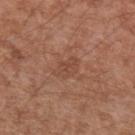Q: What are the patient's age and sex?
A: male, aged 53 to 57
Q: How was this image acquired?
A: 15 mm crop, total-body photography
Q: What did automated image analysis measure?
A: a mean CIELAB color near L≈46 a*≈22 b*≈29 and a normalized lesion–skin contrast near 5; a border-irregularity rating of about 3/10, internal color variation of about 1.5 on a 0–10 scale, and a peripheral color-asymmetry measure near 0.5; an automated nevus-likeness rating near 0 out of 100 and a detector confidence of about 100 out of 100 that the crop contains a lesion
Q: Lesion location?
A: the left upper arm
Q: Illumination type?
A: white-light illumination